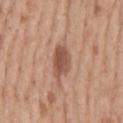workup: total-body-photography surveillance lesion; no biopsy
automated metrics: a border-irregularity index near 2/10 and a within-lesion color-variation index near 3/10
acquisition: total-body-photography crop, ~15 mm field of view
tile lighting: white-light
body site: the back
lesion diameter: ≈4 mm
patient: male, aged around 55